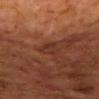Clinical impression: Part of a total-body skin-imaging series; this lesion was reviewed on a skin check and was not flagged for biopsy. Clinical summary: A male patient, aged 63–67. The recorded lesion diameter is about 2.5 mm. The lesion is located on the mid back. A 15 mm crop from a total-body photograph taken for skin-cancer surveillance.